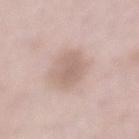follow-up: total-body-photography surveillance lesion; no biopsy | subject: male, aged 53 to 57 | lesion size: ≈5 mm | location: the mid back | tile lighting: white-light | image source: ~15 mm crop, total-body skin-cancer survey.